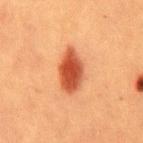The patient is a male aged 38 to 42.
Measured at roughly 5.5 mm in maximum diameter.
The tile uses cross-polarized illumination.
A 15 mm close-up extracted from a 3D total-body photography capture.
From the mid back.